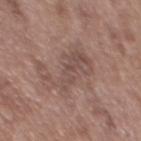The lesion was photographed on a routine skin check and not biopsied; there is no pathology result. Automated image analysis of the tile measured a footprint of about 14 mm², a shape eccentricity near 0.9, and a shape-asymmetry score of about 0.45 (0 = symmetric). The analysis additionally found a border-irregularity rating of about 7.5/10, a within-lesion color-variation index near 3.5/10, and peripheral color asymmetry of about 1. The analysis additionally found an automated nevus-likeness rating near 0 out of 100. A male patient aged 48 to 52. On the mid back. Imaged with white-light lighting. A 15 mm crop from a total-body photograph taken for skin-cancer surveillance.